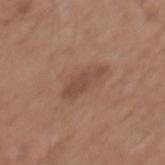Recorded during total-body skin imaging; not selected for excision or biopsy. The lesion is located on the mid back. About 4.5 mm across. A male patient, aged 63–67. Automated image analysis of the tile measured about 8 CIELAB-L* units darker than the surrounding skin and a normalized lesion–skin contrast near 6. And it measured a border-irregularity index near 5/10 and peripheral color asymmetry of about 0.5. The software also gave an automated nevus-likeness rating near 0 out of 100 and a detector confidence of about 100 out of 100 that the crop contains a lesion. Captured under white-light illumination. A 15 mm close-up tile from a total-body photography series done for melanoma screening.